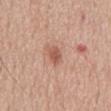Captured during whole-body skin photography for melanoma surveillance; the lesion was not biopsied. The patient is a male in their mid- to late 50s. Captured under white-light illumination. A 15 mm close-up extracted from a 3D total-body photography capture. The lesion is on the mid back. The lesion-visualizer software estimated a footprint of about 3.5 mm², an eccentricity of roughly 0.8, and two-axis asymmetry of about 0.2. The analysis additionally found about 11 CIELAB-L* units darker than the surrounding skin and a normalized border contrast of about 7. The analysis additionally found a border-irregularity rating of about 1.5/10, a color-variation rating of about 1.5/10, and radial color variation of about 0.5. About 2.5 mm across.